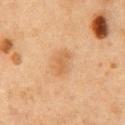{"biopsy_status": "not biopsied; imaged during a skin examination", "patient": {"sex": "male", "age_approx": 75}, "image": {"source": "total-body photography crop", "field_of_view_mm": 15}, "site": "chest"}